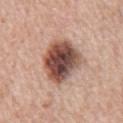workup — imaged on a skin check; not biopsied | illumination — white-light illumination | patient — male, roughly 60 years of age | anatomic site — the chest | imaging modality — total-body-photography crop, ~15 mm field of view | diameter — about 6 mm | TBP lesion metrics — an area of roughly 20 mm², a shape eccentricity near 0.65, and a symmetry-axis asymmetry near 0.15; a border-irregularity index near 1.5/10, a color-variation rating of about 8.5/10, and peripheral color asymmetry of about 2.5; an automated nevus-likeness rating near 60 out of 100 and a detector confidence of about 100 out of 100 that the crop contains a lesion.